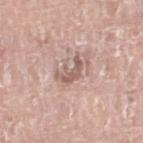notes = catalogued during a skin exam; not biopsied | acquisition = total-body-photography crop, ~15 mm field of view | lesion diameter = ~3.5 mm (longest diameter) | subject = male, aged 73–77 | lighting = white-light illumination | body site = the right lower leg | automated metrics = a lesion area of about 5.5 mm²; a mean CIELAB color near L≈59 a*≈18 b*≈24; a border-irregularity rating of about 8.5/10, internal color variation of about 2 on a 0–10 scale, and radial color variation of about 0.5.